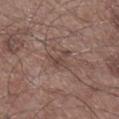workup = total-body-photography surveillance lesion; no biopsy | size = about 3 mm | subject = male, aged approximately 60 | location = the right lower leg | image = 15 mm crop, total-body photography.